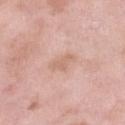Q: Was a biopsy performed?
A: imaged on a skin check; not biopsied
Q: What kind of image is this?
A: ~15 mm crop, total-body skin-cancer survey
Q: Where on the body is the lesion?
A: the right lower leg
Q: How was the tile lit?
A: white-light
Q: Who is the patient?
A: female, aged 68–72
Q: What is the lesion's diameter?
A: ≈2.5 mm
Q: Automated lesion metrics?
A: a lesion area of about 3.5 mm², a shape eccentricity near 0.75, and a shape-asymmetry score of about 0.45 (0 = symmetric); a lesion color around L≈65 a*≈21 b*≈29 in CIELAB, about 7 CIELAB-L* units darker than the surrounding skin, and a lesion-to-skin contrast of about 5 (normalized; higher = more distinct); a detector confidence of about 100 out of 100 that the crop contains a lesion